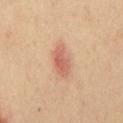Recorded during total-body skin imaging; not selected for excision or biopsy. Approximately 4 mm at its widest. The lesion is located on the chest. Captured under cross-polarized illumination. A roughly 15 mm field-of-view crop from a total-body skin photograph. The patient is a male aged approximately 55.